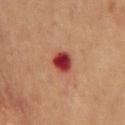The lesion was tiled from a total-body skin photograph and was not biopsied. Located on the front of the torso. A lesion tile, about 15 mm wide, cut from a 3D total-body photograph. A male subject aged 68–72.